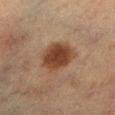Part of a total-body skin-imaging series; this lesion was reviewed on a skin check and was not flagged for biopsy. This is a cross-polarized tile. A lesion tile, about 15 mm wide, cut from a 3D total-body photograph. Automated image analysis of the tile measured a lesion area of about 14 mm², a shape eccentricity near 0.45, and a shape-asymmetry score of about 0.2 (0 = symmetric). It also reported an automated nevus-likeness rating near 100 out of 100 and lesion-presence confidence of about 100/100. About 4 mm across. Located on the left lower leg. The subject is a female about 55 years old.